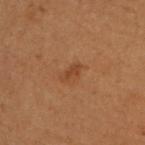A female patient aged around 50. On the upper back. The lesion's longest dimension is about 2.5 mm. Cropped from a whole-body photographic skin survey; the tile spans about 15 mm. This is a cross-polarized tile.